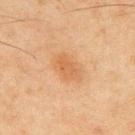workup: imaged on a skin check; not biopsied | image: 15 mm crop, total-body photography | lighting: cross-polarized illumination | site: the upper back | subject: male, about 45 years old | lesion diameter: ≈4 mm | TBP lesion metrics: a footprint of about 9 mm², an outline eccentricity of about 0.75 (0 = round, 1 = elongated), and two-axis asymmetry of about 0.2; a mean CIELAB color near L≈51 a*≈19 b*≈34, a lesion–skin lightness drop of about 6, and a normalized border contrast of about 5.5.